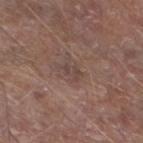| key | value |
|---|---|
| workup | total-body-photography surveillance lesion; no biopsy |
| subject | male, aged 63 to 67 |
| acquisition | total-body-photography crop, ~15 mm field of view |
| site | the leg |
| image-analysis metrics | an average lesion color of about L≈43 a*≈16 b*≈20 (CIELAB) |